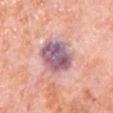{
  "biopsy_status": "not biopsied; imaged during a skin examination",
  "automated_metrics": {
    "border_irregularity_0_10": 2.0,
    "color_variation_0_10": 7.0
  },
  "image": {
    "source": "total-body photography crop",
    "field_of_view_mm": 15
  },
  "patient": {
    "sex": "male",
    "age_approx": 80
  },
  "lighting": "white-light",
  "site": "chest"
}